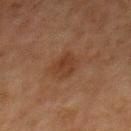Q: Is there a histopathology result?
A: imaged on a skin check; not biopsied
Q: Lesion size?
A: ~3 mm (longest diameter)
Q: Patient demographics?
A: male, in their 80s
Q: What is the imaging modality?
A: 15 mm crop, total-body photography
Q: What did automated image analysis measure?
A: a lesion area of about 5.5 mm² and an eccentricity of roughly 0.7; a nevus-likeness score of about 20/100
Q: Lesion location?
A: the front of the torso
Q: Illumination type?
A: cross-polarized